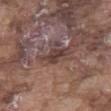Assessment:
Recorded during total-body skin imaging; not selected for excision or biopsy.
Context:
The patient is a male roughly 75 years of age. Longest diameter approximately 3.5 mm. The lesion is on the left thigh. The lesion-visualizer software estimated a symmetry-axis asymmetry near 0.25. And it measured a normalized border contrast of about 8. It also reported a nevus-likeness score of about 0/100 and a lesion-detection confidence of about 75/100. Captured under white-light illumination. Cropped from a whole-body photographic skin survey; the tile spans about 15 mm.